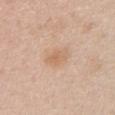This lesion was catalogued during total-body skin photography and was not selected for biopsy.
A region of skin cropped from a whole-body photographic capture, roughly 15 mm wide.
A female patient, roughly 60 years of age.
On the chest.